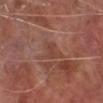{
  "biopsy_status": "not biopsied; imaged during a skin examination",
  "automated_metrics": {
    "border_irregularity_0_10": 4.5,
    "color_variation_0_10": 2.5,
    "peripheral_color_asymmetry": 1.0,
    "nevus_likeness_0_100": 0,
    "lesion_detection_confidence_0_100": 95
  },
  "lighting": "white-light",
  "patient": {
    "sex": "male",
    "age_approx": 65
  },
  "lesion_size": {
    "long_diameter_mm_approx": 3.5
  },
  "image": {
    "source": "total-body photography crop",
    "field_of_view_mm": 15
  },
  "site": "leg"
}